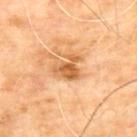Q: Is there a histopathology result?
A: total-body-photography surveillance lesion; no biopsy
Q: Where on the body is the lesion?
A: the upper back
Q: Who is the patient?
A: male, about 65 years old
Q: How was the tile lit?
A: cross-polarized
Q: What kind of image is this?
A: total-body-photography crop, ~15 mm field of view
Q: Lesion size?
A: about 3.5 mm